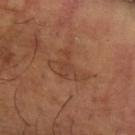biopsy status: imaged on a skin check; not biopsied | diameter: ~5 mm (longest diameter) | location: the right forearm | image source: ~15 mm tile from a whole-body skin photo | illumination: cross-polarized illumination | image-analysis metrics: an area of roughly 10 mm², an outline eccentricity of about 0.8 (0 = round, 1 = elongated), and two-axis asymmetry of about 0.55; a border-irregularity index near 6/10, internal color variation of about 3 on a 0–10 scale, and radial color variation of about 1 | patient: male, aged around 65.